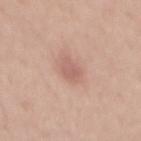notes: imaged on a skin check; not biopsied | image source: ~15 mm tile from a whole-body skin photo | site: the mid back | patient: male, roughly 30 years of age.